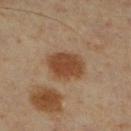  biopsy_status: not biopsied; imaged during a skin examination
  site: right lower leg
  image:
    source: total-body photography crop
    field_of_view_mm: 15
  patient:
    sex: male
    age_approx: 60
  automated_metrics:
    area_mm2_approx: 13.0
    eccentricity: 0.7
    border_irregularity_0_10: 1.5
    color_variation_0_10: 2.5
    nevus_likeness_0_100: 100
    lesion_detection_confidence_0_100: 100
  lesion_size:
    long_diameter_mm_approx: 4.5
  lighting: cross-polarized